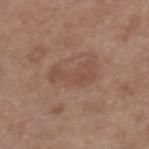Part of a total-body skin-imaging series; this lesion was reviewed on a skin check and was not flagged for biopsy. A 15 mm close-up extracted from a 3D total-body photography capture. The lesion is on the back. The patient is a female aged around 40.